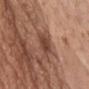• biopsy status · total-body-photography surveillance lesion; no biopsy
• imaging modality · 15 mm crop, total-body photography
• lesion diameter · ~5 mm (longest diameter)
• body site · the front of the torso
• subject · male, roughly 60 years of age